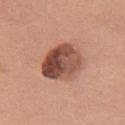Background: The lesion's longest dimension is about 6 mm. From the chest. A female subject aged around 60. A roughly 15 mm field-of-view crop from a total-body skin photograph.